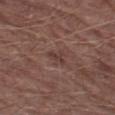No biopsy was performed on this lesion — it was imaged during a full skin examination and was not determined to be concerning.
Approximately 3 mm at its widest.
A region of skin cropped from a whole-body photographic capture, roughly 15 mm wide.
A male subject in their mid- to late 70s.
This is a white-light tile.
On the left thigh.
The lesion-visualizer software estimated an area of roughly 3.5 mm², an outline eccentricity of about 0.9 (0 = round, 1 = elongated), and a symmetry-axis asymmetry near 0.4. The software also gave a mean CIELAB color near L≈38 a*≈18 b*≈22, roughly 7 lightness units darker than nearby skin, and a lesion-to-skin contrast of about 6 (normalized; higher = more distinct).